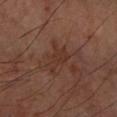site=the right forearm; patient=male, aged around 70; imaging modality=~15 mm crop, total-body skin-cancer survey.